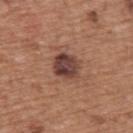notes: no biopsy performed (imaged during a skin exam)
tile lighting: white-light
image-analysis metrics: a footprint of about 8 mm², an outline eccentricity of about 0.6 (0 = round, 1 = elongated), and two-axis asymmetry of about 0.2; a lesion-detection confidence of about 100/100
patient: male, in their mid- to late 70s
anatomic site: the upper back
image source: ~15 mm tile from a whole-body skin photo